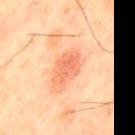follow-up = imaged on a skin check; not biopsied
acquisition = total-body-photography crop, ~15 mm field of view
subject = male, aged 43–47
location = the mid back
lesion diameter = ≈4.5 mm
tile lighting = cross-polarized illumination